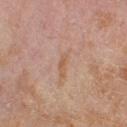• site · the right lower leg
• image · 15 mm crop, total-body photography
• patient · female, aged approximately 60
• automated metrics · a footprint of about 4 mm² and an outline eccentricity of about 0.9 (0 = round, 1 = elongated); border irregularity of about 4.5 on a 0–10 scale, internal color variation of about 2.5 on a 0–10 scale, and peripheral color asymmetry of about 0.5
• lesion size · ≈3.5 mm
• lighting · cross-polarized